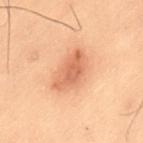{
  "biopsy_status": "not biopsied; imaged during a skin examination",
  "site": "lower back",
  "lighting": "cross-polarized",
  "image": {
    "source": "total-body photography crop",
    "field_of_view_mm": 15
  },
  "patient": {
    "sex": "male",
    "age_approx": 55
  },
  "lesion_size": {
    "long_diameter_mm_approx": 5.0
  }
}